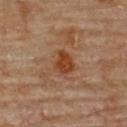biopsy_status: not biopsied; imaged during a skin examination